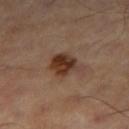Imaged during a routine full-body skin examination; the lesion was not biopsied and no histopathology is available.
A 15 mm close-up extracted from a 3D total-body photography capture.
The lesion is located on the right thigh.
Captured under cross-polarized illumination.
Measured at roughly 3.5 mm in maximum diameter.